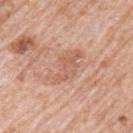Imaged during a routine full-body skin examination; the lesion was not biopsied and no histopathology is available. Automated image analysis of the tile measured a nevus-likeness score of about 0/100. Captured under white-light illumination. A 15 mm crop from a total-body photograph taken for skin-cancer surveillance. Located on the left upper arm. The subject is a male aged around 80.